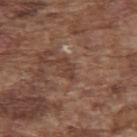notes: total-body-photography surveillance lesion; no biopsy | location: the upper back | imaging modality: 15 mm crop, total-body photography | tile lighting: white-light illumination | size: ~2.5 mm (longest diameter) | automated lesion analysis: an area of roughly 2.5 mm² and an outline eccentricity of about 0.85 (0 = round, 1 = elongated); a lesion–skin lightness drop of about 7 and a normalized border contrast of about 6; a lesion-detection confidence of about 55/100 | subject: male, aged 73–77.